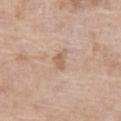Clinical impression:
Imaged during a routine full-body skin examination; the lesion was not biopsied and no histopathology is available.